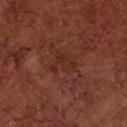follow-up: imaged on a skin check; not biopsied
automated metrics: a lesion area of about 5 mm², an outline eccentricity of about 0.7 (0 = round, 1 = elongated), and a shape-asymmetry score of about 0.6 (0 = symmetric); a lesion color around L≈27 a*≈24 b*≈26 in CIELAB, about 4 CIELAB-L* units darker than the surrounding skin, and a normalized border contrast of about 4.5
image: ~15 mm crop, total-body skin-cancer survey
body site: the arm
patient: male, aged 63–67